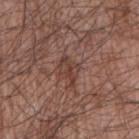Recorded during total-body skin imaging; not selected for excision or biopsy.
Located on the right upper arm.
Measured at roughly 3.5 mm in maximum diameter.
Imaged with white-light lighting.
A male patient, aged approximately 65.
Cropped from a total-body skin-imaging series; the visible field is about 15 mm.